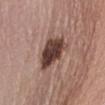patient: female, aged around 65; site: the front of the torso; imaging modality: total-body-photography crop, ~15 mm field of view.